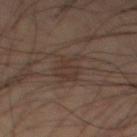biopsy status: imaged on a skin check; not biopsied | image-analysis metrics: an area of roughly 7.5 mm², an eccentricity of roughly 0.8, and a symmetry-axis asymmetry near 0.3; border irregularity of about 5.5 on a 0–10 scale and peripheral color asymmetry of about 1 | image source: total-body-photography crop, ~15 mm field of view | lesion diameter: about 4.5 mm | tile lighting: cross-polarized | location: the leg | subject: male, aged approximately 60.